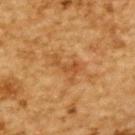{"biopsy_status": "not biopsied; imaged during a skin examination", "site": "upper back", "automated_metrics": {"cielab_L": 44, "cielab_a": 22, "cielab_b": 38, "vs_skin_darker_L": 7.0, "nevus_likeness_0_100": 0, "lesion_detection_confidence_0_100": 100}, "image": {"source": "total-body photography crop", "field_of_view_mm": 15}, "lighting": "cross-polarized", "lesion_size": {"long_diameter_mm_approx": 3.5}, "patient": {"sex": "male", "age_approx": "85+"}}